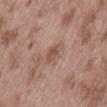Impression: This lesion was catalogued during total-body skin photography and was not selected for biopsy. Clinical summary: Located on the lower back. Captured under white-light illumination. The recorded lesion diameter is about 3 mm. A close-up tile cropped from a whole-body skin photograph, about 15 mm across. The patient is a male aged around 55.